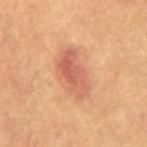Q: Was this lesion biopsied?
A: no biopsy performed (imaged during a skin exam)
Q: How was the tile lit?
A: cross-polarized
Q: What kind of image is this?
A: ~15 mm tile from a whole-body skin photo
Q: Who is the patient?
A: male, roughly 55 years of age
Q: Lesion location?
A: the back